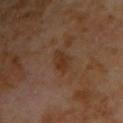No biopsy was performed on this lesion — it was imaged during a full skin examination and was not determined to be concerning.
The lesion is on the right upper arm.
The patient is a male aged 58–62.
A 15 mm close-up tile from a total-body photography series done for melanoma screening.